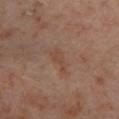Recorded during total-body skin imaging; not selected for excision or biopsy.
Longest diameter approximately 3 mm.
The lesion-visualizer software estimated a lesion-detection confidence of about 100/100.
The patient is a male about 70 years old.
The lesion is located on the left lower leg.
A region of skin cropped from a whole-body photographic capture, roughly 15 mm wide.